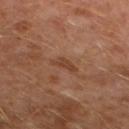Assessment: Imaged during a routine full-body skin examination; the lesion was not biopsied and no histopathology is available. Acquisition and patient details: The lesion's longest dimension is about 3 mm. A roughly 15 mm field-of-view crop from a total-body skin photograph. The lesion is on the left lower leg. The patient is a male aged 28 to 32.